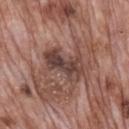notes — imaged on a skin check; not biopsied | TBP lesion metrics — a lesion color around L≈41 a*≈19 b*≈21 in CIELAB, about 11 CIELAB-L* units darker than the surrounding skin, and a lesion-to-skin contrast of about 9 (normalized; higher = more distinct); a nevus-likeness score of about 0/100 and a detector confidence of about 100 out of 100 that the crop contains a lesion | image source — ~15 mm tile from a whole-body skin photo | body site — the mid back | lesion diameter — about 5.5 mm | subject — male, aged 68–72.